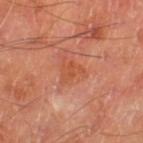Impression: Imaged during a routine full-body skin examination; the lesion was not biopsied and no histopathology is available. Acquisition and patient details: The lesion is located on the left thigh. Approximately 3 mm at its widest. An algorithmic analysis of the crop reported an automated nevus-likeness rating near 0 out of 100 and a detector confidence of about 100 out of 100 that the crop contains a lesion. A male subject aged 68–72. A 15 mm crop from a total-body photograph taken for skin-cancer surveillance.